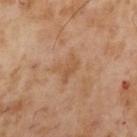Notes:
• biopsy status · total-body-photography surveillance lesion; no biopsy
• imaging modality · 15 mm crop, total-body photography
• size · ≈3 mm
• subject · male, aged around 55
• tile lighting · cross-polarized
• body site · the left upper arm